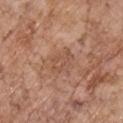Captured during whole-body skin photography for melanoma surveillance; the lesion was not biopsied. The recorded lesion diameter is about 3.5 mm. The lesion is on the chest. Cropped from a total-body skin-imaging series; the visible field is about 15 mm. A male patient aged around 70. Imaged with white-light lighting.